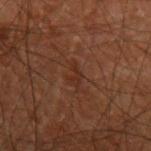Clinical impression:
The lesion was tiled from a total-body skin photograph and was not biopsied.
Clinical summary:
A close-up tile cropped from a whole-body skin photograph, about 15 mm across. On the arm. A male patient, aged approximately 70.